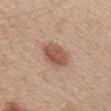biopsy status: no biopsy performed (imaged during a skin exam); tile lighting: white-light illumination; body site: the head or neck; subject: female, aged 38 to 42; lesion size: about 4 mm; acquisition: ~15 mm tile from a whole-body skin photo.